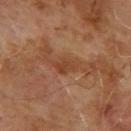{
  "biopsy_status": "not biopsied; imaged during a skin examination",
  "automated_metrics": {
    "area_mm2_approx": 4.5,
    "eccentricity": 0.85,
    "nevus_likeness_0_100": 0,
    "lesion_detection_confidence_0_100": 100
  },
  "image": {
    "source": "total-body photography crop",
    "field_of_view_mm": 15
  },
  "lesion_size": {
    "long_diameter_mm_approx": 3.5
  },
  "site": "upper back",
  "patient": {
    "sex": "male",
    "age_approx": 65
  },
  "lighting": "cross-polarized"
}